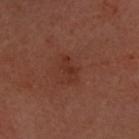This lesion was catalogued during total-body skin photography and was not selected for biopsy.
A 15 mm crop from a total-body photograph taken for skin-cancer surveillance.
The subject is a female about 60 years old.
The lesion's longest dimension is about 3 mm.
The lesion is located on the head or neck.